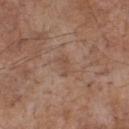workup: total-body-photography surveillance lesion; no biopsy | site: the chest | tile lighting: white-light illumination | size: ~2.5 mm (longest diameter) | image source: ~15 mm crop, total-body skin-cancer survey | patient: male, about 70 years old.